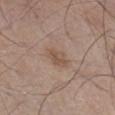Q: What is the imaging modality?
A: 15 mm crop, total-body photography
Q: Who is the patient?
A: male, roughly 45 years of age
Q: Lesion location?
A: the right lower leg